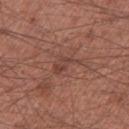Imaged during a routine full-body skin examination; the lesion was not biopsied and no histopathology is available. This is a white-light tile. The total-body-photography lesion software estimated an outline eccentricity of about 0.85 (0 = round, 1 = elongated) and a symmetry-axis asymmetry near 0.45. And it measured a color-variation rating of about 2/10. The analysis additionally found an automated nevus-likeness rating near 0 out of 100 and lesion-presence confidence of about 100/100. A male subject, aged around 55. A 15 mm crop from a total-body photograph taken for skin-cancer surveillance. On the leg.